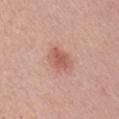Findings:
– workup: imaged on a skin check; not biopsied
– TBP lesion metrics: a footprint of about 6.5 mm² and a symmetry-axis asymmetry near 0.25; an average lesion color of about L≈58 a*≈25 b*≈28 (CIELAB) and a normalized lesion–skin contrast near 6.5; border irregularity of about 2.5 on a 0–10 scale, internal color variation of about 2.5 on a 0–10 scale, and a peripheral color-asymmetry measure near 0.5
– lesion size: ~3.5 mm (longest diameter)
– location: the arm
– patient: male, aged around 55
– image source: ~15 mm crop, total-body skin-cancer survey
– tile lighting: white-light